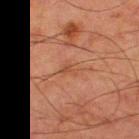This lesion was catalogued during total-body skin photography and was not selected for biopsy. The patient is a male approximately 80 years of age. The recorded lesion diameter is about 3 mm. The lesion is on the right thigh. A 15 mm close-up extracted from a 3D total-body photography capture. The lesion-visualizer software estimated a footprint of about 4 mm² and an outline eccentricity of about 0.75 (0 = round, 1 = elongated). It also reported a within-lesion color-variation index near 2/10. The analysis additionally found an automated nevus-likeness rating near 0 out of 100 and a detector confidence of about 95 out of 100 that the crop contains a lesion. Imaged with cross-polarized lighting.